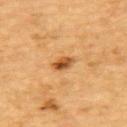Clinical impression: Imaged during a routine full-body skin examination; the lesion was not biopsied and no histopathology is available. Background: A male patient, roughly 85 years of age or older. From the upper back. A region of skin cropped from a whole-body photographic capture, roughly 15 mm wide.